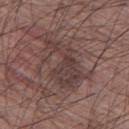Q: Is there a histopathology result?
A: catalogued during a skin exam; not biopsied